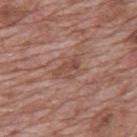{"biopsy_status": "not biopsied; imaged during a skin examination", "automated_metrics": {"area_mm2_approx": 5.0, "eccentricity": 0.8, "shape_asymmetry": 0.45, "cielab_L": 48, "cielab_a": 21, "cielab_b": 27, "vs_skin_darker_L": 8.0, "vs_skin_contrast_norm": 6.0, "border_irregularity_0_10": 5.5, "color_variation_0_10": 3.0, "peripheral_color_asymmetry": 1.0}, "lesion_size": {"long_diameter_mm_approx": 3.5}, "lighting": "white-light", "image": {"source": "total-body photography crop", "field_of_view_mm": 15}, "patient": {"sex": "male", "age_approx": 70}, "site": "back"}